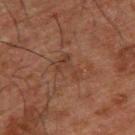notes: no biopsy performed (imaged during a skin exam); diameter: ≈3.5 mm; site: the upper back; image source: 15 mm crop, total-body photography; subject: male, aged approximately 50; tile lighting: cross-polarized illumination.